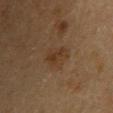Assessment: Recorded during total-body skin imaging; not selected for excision or biopsy. Background: Captured under cross-polarized illumination. A 15 mm close-up extracted from a 3D total-body photography capture. The subject is a male in their 60s. The total-body-photography lesion software estimated border irregularity of about 3 on a 0–10 scale, a within-lesion color-variation index near 3/10, and peripheral color asymmetry of about 1. The software also gave a lesion-detection confidence of about 100/100. Longest diameter approximately 3.5 mm.